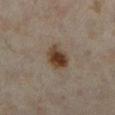A 15 mm close-up extracted from a 3D total-body photography capture.
A female patient aged 33–37.
Located on the left lower leg.
The lesion's longest dimension is about 3.5 mm.
Automated image analysis of the tile measured an area of roughly 8 mm² and a shape eccentricity near 0.7. It also reported a lesion color around L≈38 a*≈14 b*≈26 in CIELAB and a normalized lesion–skin contrast near 11.